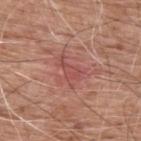Captured during whole-body skin photography for melanoma surveillance; the lesion was not biopsied. A male patient about 60 years old. Located on the upper back. A 15 mm close-up tile from a total-body photography series done for melanoma screening. The lesion-visualizer software estimated a lesion area of about 4 mm², a shape eccentricity near 0.85, and a shape-asymmetry score of about 0.5 (0 = symmetric). It also reported an automated nevus-likeness rating near 0 out of 100 and a detector confidence of about 90 out of 100 that the crop contains a lesion. The lesion's longest dimension is about 3 mm. Captured under white-light illumination.